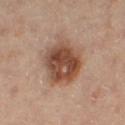Clinical impression: Recorded during total-body skin imaging; not selected for excision or biopsy. Acquisition and patient details: The lesion is on the right thigh. A female patient approximately 45 years of age. The recorded lesion diameter is about 5.5 mm. A region of skin cropped from a whole-body photographic capture, roughly 15 mm wide. The tile uses cross-polarized illumination.